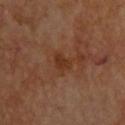| key | value |
|---|---|
| follow-up | total-body-photography surveillance lesion; no biopsy |
| location | the upper back |
| lighting | cross-polarized |
| TBP lesion metrics | a footprint of about 4 mm², an outline eccentricity of about 0.7 (0 = round, 1 = elongated), and a shape-asymmetry score of about 0.3 (0 = symmetric); a lesion color around L≈32 a*≈21 b*≈31 in CIELAB, roughly 6 lightness units darker than nearby skin, and a normalized lesion–skin contrast near 6.5; a classifier nevus-likeness of about 0/100 and a lesion-detection confidence of about 100/100 |
| patient | male, approximately 60 years of age |
| image source | ~15 mm crop, total-body skin-cancer survey |